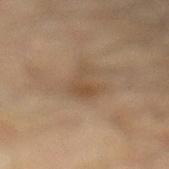The lesion was tiled from a total-body skin photograph and was not biopsied.
Cropped from a whole-body photographic skin survey; the tile spans about 15 mm.
The lesion is on the left lower leg.
Automated image analysis of the tile measured an average lesion color of about L≈45 a*≈14 b*≈29 (CIELAB) and a lesion–skin lightness drop of about 7. And it measured a nevus-likeness score of about 0/100 and lesion-presence confidence of about 100/100.
A male subject, aged 63–67.